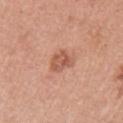Impression:
Imaged during a routine full-body skin examination; the lesion was not biopsied and no histopathology is available.
Clinical summary:
Cropped from a whole-body photographic skin survey; the tile spans about 15 mm. The tile uses white-light illumination. The total-body-photography lesion software estimated a lesion color around L≈56 a*≈25 b*≈31 in CIELAB, a lesion–skin lightness drop of about 10, and a lesion-to-skin contrast of about 7 (normalized; higher = more distinct). It also reported a border-irregularity rating of about 3.5/10, a within-lesion color-variation index near 4/10, and peripheral color asymmetry of about 1.5. The analysis additionally found a classifier nevus-likeness of about 20/100 and a lesion-detection confidence of about 100/100. A female patient, in their mid- to late 30s. The lesion is on the arm. The recorded lesion diameter is about 3 mm.